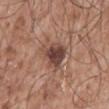Clinical impression: The lesion was tiled from a total-body skin photograph and was not biopsied. Background: A male subject in their mid- to late 50s. The lesion is on the mid back. A close-up tile cropped from a whole-body skin photograph, about 15 mm across. The total-body-photography lesion software estimated a lesion area of about 10 mm² and a shape eccentricity near 0.7. The software also gave an average lesion color of about L≈44 a*≈20 b*≈24 (CIELAB) and a normalized lesion–skin contrast near 9.5. And it measured a border-irregularity rating of about 3.5/10 and a within-lesion color-variation index near 5.5/10. It also reported a lesion-detection confidence of about 100/100. The recorded lesion diameter is about 4.5 mm.